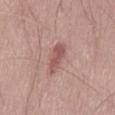biopsy status: no biopsy performed (imaged during a skin exam)
diameter: ~4.5 mm (longest diameter)
image-analysis metrics: a lesion area of about 6 mm² and an eccentricity of roughly 0.95; a lesion color around L≈53 a*≈23 b*≈23 in CIELAB, about 11 CIELAB-L* units darker than the surrounding skin, and a lesion-to-skin contrast of about 7 (normalized; higher = more distinct); a nevus-likeness score of about 10/100 and lesion-presence confidence of about 100/100
location: the left thigh
imaging modality: 15 mm crop, total-body photography
tile lighting: white-light
subject: male, in their 50s